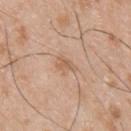notes=no biopsy performed (imaged during a skin exam)
image=15 mm crop, total-body photography
size=≈2.5 mm
patient=male, in their mid-40s
site=the upper back